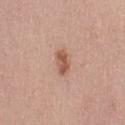This lesion was catalogued during total-body skin photography and was not selected for biopsy. Longest diameter approximately 3 mm. A female subject aged around 45. The tile uses white-light illumination. From the left thigh. A lesion tile, about 15 mm wide, cut from a 3D total-body photograph.